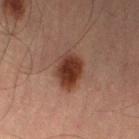| field | value |
|---|---|
| notes | catalogued during a skin exam; not biopsied |
| automated metrics | an average lesion color of about L≈30 a*≈20 b*≈24 (CIELAB) and roughly 13 lightness units darker than nearby skin; border irregularity of about 2 on a 0–10 scale, internal color variation of about 3.5 on a 0–10 scale, and radial color variation of about 1 |
| subject | male, aged 53–57 |
| tile lighting | cross-polarized |
| acquisition | 15 mm crop, total-body photography |
| anatomic site | the leg |